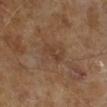No biopsy was performed on this lesion — it was imaged during a full skin examination and was not determined to be concerning. The subject is a male aged 63 to 67. This is a cross-polarized tile. Cropped from a whole-body photographic skin survey; the tile spans about 15 mm. The recorded lesion diameter is about 3 mm.